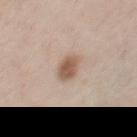{"biopsy_status": "not biopsied; imaged during a skin examination", "image": {"source": "total-body photography crop", "field_of_view_mm": 15}, "lesion_size": {"long_diameter_mm_approx": 2.5}, "lighting": "white-light", "site": "front of the torso", "patient": {"sex": "female", "age_approx": 55}, "automated_metrics": {"area_mm2_approx": 5.5, "eccentricity": 0.5, "cielab_L": 56, "cielab_a": 18, "cielab_b": 28, "vs_skin_darker_L": 13.0, "vs_skin_contrast_norm": 8.5, "border_irregularity_0_10": 2.0, "color_variation_0_10": 2.5, "peripheral_color_asymmetry": 1.0, "nevus_likeness_0_100": 95, "lesion_detection_confidence_0_100": 100}}